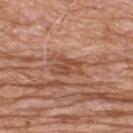notes: total-body-photography surveillance lesion; no biopsy
lesion size: ≈4 mm
patient: male, aged approximately 80
lighting: white-light
imaging modality: ~15 mm tile from a whole-body skin photo
anatomic site: the upper back